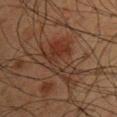Captured during whole-body skin photography for melanoma surveillance; the lesion was not biopsied.
This is a cross-polarized tile.
Measured at roughly 6 mm in maximum diameter.
A male patient aged approximately 60.
This image is a 15 mm lesion crop taken from a total-body photograph.
The lesion is on the chest.
The lesion-visualizer software estimated an automated nevus-likeness rating near 0 out of 100 and lesion-presence confidence of about 90/100.